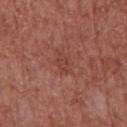Findings:
– workup · imaged on a skin check; not biopsied
– image · ~15 mm crop, total-body skin-cancer survey
– size · ~3 mm (longest diameter)
– automated lesion analysis · a nevus-likeness score of about 0/100 and a lesion-detection confidence of about 100/100
– site · the abdomen
– subject · male, aged 63–67